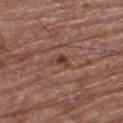Recorded during total-body skin imaging; not selected for excision or biopsy. The total-body-photography lesion software estimated a mean CIELAB color near L≈41 a*≈20 b*≈24, about 8 CIELAB-L* units darker than the surrounding skin, and a lesion-to-skin contrast of about 7 (normalized; higher = more distinct). And it measured a border-irregularity index near 5/10, internal color variation of about 2 on a 0–10 scale, and a peripheral color-asymmetry measure near 0.5. It also reported lesion-presence confidence of about 100/100. A male subject, approximately 65 years of age. Captured under white-light illumination. A 15 mm crop from a total-body photograph taken for skin-cancer surveillance. Approximately 2.5 mm at its widest. The lesion is located on the right thigh.